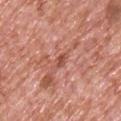Findings:
• follow-up — no biopsy performed (imaged during a skin exam)
• lighting — white-light illumination
• lesion size — about 2.5 mm
• image — 15 mm crop, total-body photography
• body site — the chest
• automated lesion analysis — a footprint of about 2.5 mm², a shape eccentricity near 0.9, and a shape-asymmetry score of about 0.3 (0 = symmetric); about 9 CIELAB-L* units darker than the surrounding skin and a normalized border contrast of about 7; a classifier nevus-likeness of about 0/100 and a detector confidence of about 85 out of 100 that the crop contains a lesion
• subject — male, approximately 75 years of age